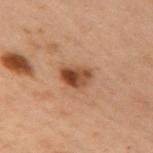Assessment: No biopsy was performed on this lesion — it was imaged during a full skin examination and was not determined to be concerning. Background: A female patient aged 53–57. This image is a 15 mm lesion crop taken from a total-body photograph. The tile uses cross-polarized illumination. The lesion is on the left arm. The lesion-visualizer software estimated a lesion area of about 6 mm², an eccentricity of roughly 0.75, and a symmetry-axis asymmetry near 0.3. The software also gave a mean CIELAB color near L≈38 a*≈20 b*≈29, about 12 CIELAB-L* units darker than the surrounding skin, and a normalized border contrast of about 10.5. And it measured a nevus-likeness score of about 95/100. Approximately 3 mm at its widest.